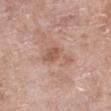biopsy status — no biopsy performed (imaged during a skin exam) | subject — female, aged 73 to 77 | location — the chest | imaging modality — 15 mm crop, total-body photography.